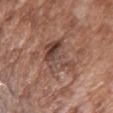biopsy status: no biopsy performed (imaged during a skin exam) | illumination: white-light | anatomic site: the chest | lesion size: ≈4.5 mm | imaging modality: total-body-photography crop, ~15 mm field of view | TBP lesion metrics: a footprint of about 13 mm² and an eccentricity of roughly 0.45; a lesion color around L≈45 a*≈19 b*≈24 in CIELAB and about 9 CIELAB-L* units darker than the surrounding skin; border irregularity of about 5.5 on a 0–10 scale, internal color variation of about 9 on a 0–10 scale, and a peripheral color-asymmetry measure near 3 | subject: female, in their mid- to late 70s.